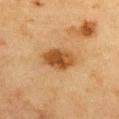Imaged during a routine full-body skin examination; the lesion was not biopsied and no histopathology is available. The lesion is located on the chest. About 4.5 mm across. Automated tile analysis of the lesion measured a footprint of about 10 mm², a shape eccentricity near 0.8, and a shape-asymmetry score of about 0.15 (0 = symmetric). And it measured a lesion–skin lightness drop of about 11 and a normalized lesion–skin contrast near 9.5. The analysis additionally found an automated nevus-likeness rating near 95 out of 100 and lesion-presence confidence of about 100/100. A lesion tile, about 15 mm wide, cut from a 3D total-body photograph. Imaged with cross-polarized lighting. A female patient about 55 years old.